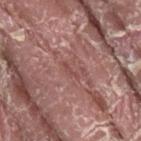| key | value |
|---|---|
| notes | imaged on a skin check; not biopsied |
| site | the right thigh |
| tile lighting | white-light |
| lesion size | ~2.5 mm (longest diameter) |
| image | total-body-photography crop, ~15 mm field of view |
| TBP lesion metrics | a footprint of about 2 mm², an eccentricity of roughly 0.95, and a shape-asymmetry score of about 0.5 (0 = symmetric); a border-irregularity rating of about 5.5/10 and a within-lesion color-variation index near 0/10; lesion-presence confidence of about 65/100 |
| patient | male, roughly 40 years of age |